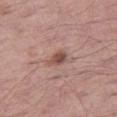notes — total-body-photography surveillance lesion; no biopsy | anatomic site — the right thigh | automated lesion analysis — a classifier nevus-likeness of about 70/100 | acquisition — ~15 mm crop, total-body skin-cancer survey | lighting — white-light illumination | patient — male, aged approximately 65 | lesion size — ~2.5 mm (longest diameter).